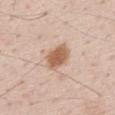Q: Was this lesion biopsied?
A: catalogued during a skin exam; not biopsied
Q: How large is the lesion?
A: ≈4.5 mm
Q: Patient demographics?
A: male, aged 48–52
Q: How was this image acquired?
A: ~15 mm tile from a whole-body skin photo
Q: Where on the body is the lesion?
A: the upper back
Q: What did automated image analysis measure?
A: a border-irregularity index near 3/10 and internal color variation of about 3 on a 0–10 scale
Q: What lighting was used for the tile?
A: white-light illumination